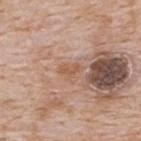Notes:
– notes — catalogued during a skin exam; not biopsied
– automated lesion analysis — an area of roughly 3.5 mm² and a shape-asymmetry score of about 0.35 (0 = symmetric); a mean CIELAB color near L≈56 a*≈21 b*≈31; border irregularity of about 3.5 on a 0–10 scale, a color-variation rating of about 2/10, and a peripheral color-asymmetry measure near 0.5; a nevus-likeness score of about 0/100
– anatomic site — the upper back
– lesion diameter — ~3 mm (longest diameter)
– subject — male, aged approximately 65
– image source — ~15 mm tile from a whole-body skin photo
– illumination — white-light illumination